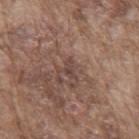Q: Was this lesion biopsied?
A: catalogued during a skin exam; not biopsied
Q: What is the lesion's diameter?
A: ≈2.5 mm
Q: How was this image acquired?
A: ~15 mm crop, total-body skin-cancer survey
Q: Where on the body is the lesion?
A: the mid back
Q: Patient demographics?
A: male, roughly 75 years of age
Q: What did automated image analysis measure?
A: a nevus-likeness score of about 0/100 and a detector confidence of about 70 out of 100 that the crop contains a lesion